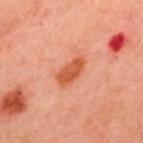- workup · no biopsy performed (imaged during a skin exam)
- lesion diameter · about 4 mm
- patient · male, approximately 50 years of age
- TBP lesion metrics · a footprint of about 7 mm², an outline eccentricity of about 0.85 (0 = round, 1 = elongated), and two-axis asymmetry of about 0.2; an average lesion color of about L≈56 a*≈33 b*≈39 (CIELAB), about 11 CIELAB-L* units darker than the surrounding skin, and a lesion-to-skin contrast of about 8.5 (normalized; higher = more distinct); a border-irregularity index near 2.5/10, internal color variation of about 2 on a 0–10 scale, and radial color variation of about 0.5
- image · ~15 mm tile from a whole-body skin photo
- illumination · cross-polarized illumination
- site · the upper back